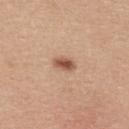Recorded during total-body skin imaging; not selected for excision or biopsy.
The lesion is on the back.
A female subject, in their mid- to late 30s.
Captured under white-light illumination.
Approximately 3 mm at its widest.
A lesion tile, about 15 mm wide, cut from a 3D total-body photograph.